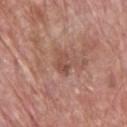The lesion was tiled from a total-body skin photograph and was not biopsied.
A lesion tile, about 15 mm wide, cut from a 3D total-body photograph.
The subject is a male aged approximately 65.
The lesion is located on the chest.